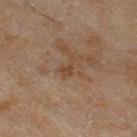<case>
  <biopsy_status>not biopsied; imaged during a skin examination</biopsy_status>
  <site>right thigh</site>
  <automated_metrics>
    <area_mm2_approx>2.5</area_mm2_approx>
    <shape_asymmetry>0.6</shape_asymmetry>
    <border_irregularity_0_10>6.0</border_irregularity_0_10>
    <lesion_detection_confidence_0_100>100</lesion_detection_confidence_0_100>
  </automated_metrics>
  <lesion_size>
    <long_diameter_mm_approx>2.5</long_diameter_mm_approx>
  </lesion_size>
  <image>
    <source>total-body photography crop</source>
    <field_of_view_mm>15</field_of_view_mm>
  </image>
  <lighting>cross-polarized</lighting>
  <patient>
    <sex>female</sex>
    <age_approx>60</age_approx>
  </patient>
</case>